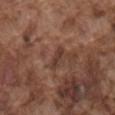- follow-up · total-body-photography surveillance lesion; no biopsy
- image source · total-body-photography crop, ~15 mm field of view
- body site · the mid back
- subject · male, in their mid-70s
- lighting · white-light illumination
- automated metrics · a normalized border contrast of about 7; a detector confidence of about 90 out of 100 that the crop contains a lesion
- lesion size · about 3 mm